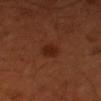follow-up = imaged on a skin check; not biopsied
site = the right upper arm
acquisition = ~15 mm crop, total-body skin-cancer survey
patient = male, about 50 years old